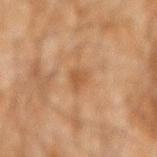Assessment:
Imaged during a routine full-body skin examination; the lesion was not biopsied and no histopathology is available.
Background:
This is a cross-polarized tile. Automated tile analysis of the lesion measured a mean CIELAB color near L≈43 a*≈18 b*≈31 and a lesion-to-skin contrast of about 5.5 (normalized; higher = more distinct). And it measured an automated nevus-likeness rating near 5 out of 100 and a lesion-detection confidence of about 100/100. Cropped from a total-body skin-imaging series; the visible field is about 15 mm. A male patient, aged 43–47. Longest diameter approximately 2.5 mm. The lesion is on the left forearm.